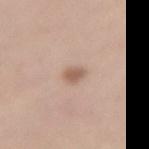workup=no biopsy performed (imaged during a skin exam) | image=~15 mm tile from a whole-body skin photo | tile lighting=white-light illumination | patient=female, roughly 45 years of age | site=the lower back | lesion size=≈2.5 mm.